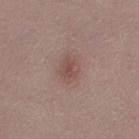Assessment: Captured during whole-body skin photography for melanoma surveillance; the lesion was not biopsied. Acquisition and patient details: The patient is a female aged 28 to 32. Captured under white-light illumination. An algorithmic analysis of the crop reported an average lesion color of about L≈49 a*≈19 b*≈22 (CIELAB), about 8 CIELAB-L* units darker than the surrounding skin, and a normalized border contrast of about 6. The analysis additionally found a border-irregularity index near 2.5/10 and a peripheral color-asymmetry measure near 0.5. On the right thigh. A close-up tile cropped from a whole-body skin photograph, about 15 mm across.